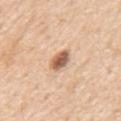workup: catalogued during a skin exam; not biopsied | subject: male, aged 68–72 | location: the mid back | imaging modality: ~15 mm crop, total-body skin-cancer survey | lesion diameter: about 3 mm | illumination: white-light | automated lesion analysis: a lesion color around L≈60 a*≈21 b*≈32 in CIELAB, a lesion–skin lightness drop of about 17, and a lesion-to-skin contrast of about 10 (normalized; higher = more distinct); a color-variation rating of about 5.5/10.